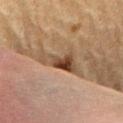notes: total-body-photography surveillance lesion; no biopsy